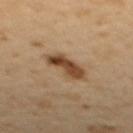  biopsy_status: not biopsied; imaged during a skin examination
  lesion_size:
    long_diameter_mm_approx: 4.5
  image:
    source: total-body photography crop
    field_of_view_mm: 15
  patient:
    sex: female
    age_approx: 55
  lighting: cross-polarized
  site: back